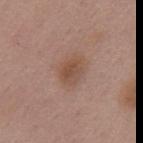This lesion was catalogued during total-body skin photography and was not selected for biopsy. From the back. Cropped from a whole-body photographic skin survey; the tile spans about 15 mm. The total-body-photography lesion software estimated internal color variation of about 3.5 on a 0–10 scale. And it measured a nevus-likeness score of about 20/100 and lesion-presence confidence of about 100/100. Longest diameter approximately 3.5 mm. A female subject about 50 years old.